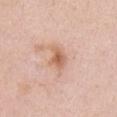The lesion was tiled from a total-body skin photograph and was not biopsied.
A roughly 15 mm field-of-view crop from a total-body skin photograph.
The recorded lesion diameter is about 3.5 mm.
Automated tile analysis of the lesion measured a lesion area of about 5 mm², a shape eccentricity near 0.75, and a symmetry-axis asymmetry near 0.25. And it measured a lesion–skin lightness drop of about 11 and a lesion-to-skin contrast of about 7.5 (normalized; higher = more distinct). And it measured a border-irregularity index near 3/10, internal color variation of about 2.5 on a 0–10 scale, and radial color variation of about 0.5. The software also gave an automated nevus-likeness rating near 80 out of 100.
The lesion is located on the chest.
The patient is a female roughly 35 years of age.
Imaged with white-light lighting.